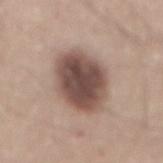The lesion was photographed on a routine skin check and not biopsied; there is no pathology result. The lesion is located on the mid back. Approximately 6.5 mm at its widest. The tile uses white-light illumination. Automated tile analysis of the lesion measured a footprint of about 24 mm², an outline eccentricity of about 0.65 (0 = round, 1 = elongated), and a symmetry-axis asymmetry near 0.15. The software also gave an average lesion color of about L≈49 a*≈17 b*≈22 (CIELAB), a lesion–skin lightness drop of about 17, and a normalized lesion–skin contrast near 11.5. It also reported a classifier nevus-likeness of about 40/100 and a lesion-detection confidence of about 100/100. A male patient, aged around 45. A region of skin cropped from a whole-body photographic capture, roughly 15 mm wide.